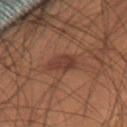No biopsy was performed on this lesion — it was imaged during a full skin examination and was not determined to be concerning.
Measured at roughly 3.5 mm in maximum diameter.
A 15 mm crop from a total-body photograph taken for skin-cancer surveillance.
A male patient in their mid- to late 50s.
Imaged with cross-polarized lighting.
On the leg.